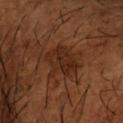Clinical impression: The lesion was photographed on a routine skin check and not biopsied; there is no pathology result. Background: A 15 mm close-up extracted from a 3D total-body photography capture. The patient is a male about 65 years old. About 4.5 mm across. The tile uses cross-polarized illumination. The lesion is on the right forearm. Automated tile analysis of the lesion measured an average lesion color of about L≈27 a*≈21 b*≈28 (CIELAB), a lesion–skin lightness drop of about 7, and a lesion-to-skin contrast of about 7.5 (normalized; higher = more distinct). It also reported a border-irregularity rating of about 5/10, a color-variation rating of about 4/10, and a peripheral color-asymmetry measure near 1.